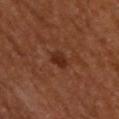{
  "biopsy_status": "not biopsied; imaged during a skin examination",
  "site": "upper back",
  "patient": {
    "sex": "male",
    "age_approx": 65
  },
  "lesion_size": {
    "long_diameter_mm_approx": 2.5
  },
  "lighting": "cross-polarized",
  "image": {
    "source": "total-body photography crop",
    "field_of_view_mm": 15
  }
}